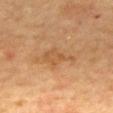The tile uses cross-polarized illumination.
The subject is a female about 55 years old.
Longest diameter approximately 5 mm.
The lesion is on the mid back.
A 15 mm crop from a total-body photograph taken for skin-cancer surveillance.